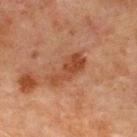notes: imaged on a skin check; not biopsied | patient: male, roughly 65 years of age | diameter: about 5 mm | tile lighting: cross-polarized illumination | image source: ~15 mm crop, total-body skin-cancer survey | anatomic site: the chest.